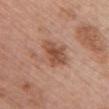Assessment: Captured during whole-body skin photography for melanoma surveillance; the lesion was not biopsied. Acquisition and patient details: A female subject aged around 60. Measured at roughly 4.5 mm in maximum diameter. From the chest. This is a white-light tile. Automated image analysis of the tile measured a lesion area of about 11 mm², an outline eccentricity of about 0.65 (0 = round, 1 = elongated), and a symmetry-axis asymmetry near 0.25. The analysis additionally found an average lesion color of about L≈52 a*≈22 b*≈30 (CIELAB), about 10 CIELAB-L* units darker than the surrounding skin, and a normalized lesion–skin contrast near 7. The analysis additionally found border irregularity of about 3.5 on a 0–10 scale and internal color variation of about 4.5 on a 0–10 scale. A 15 mm close-up extracted from a 3D total-body photography capture.